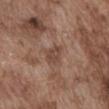Part of a total-body skin-imaging series; this lesion was reviewed on a skin check and was not flagged for biopsy.
From the abdomen.
About 3 mm across.
A male patient roughly 75 years of age.
A roughly 15 mm field-of-view crop from a total-body skin photograph.
Captured under white-light illumination.